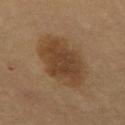<record>
  <biopsy_status>not biopsied; imaged during a skin examination</biopsy_status>
  <patient>
    <sex>male</sex>
    <age_approx>55</age_approx>
  </patient>
  <site>mid back</site>
  <lighting>cross-polarized</lighting>
  <image>
    <source>total-body photography crop</source>
    <field_of_view_mm>15</field_of_view_mm>
  </image>
  <lesion_size>
    <long_diameter_mm_approx>7.5</long_diameter_mm_approx>
  </lesion_size>
  <automated_metrics>
    <cielab_L>39</cielab_L>
    <cielab_a>16</cielab_a>
    <cielab_b>30</cielab_b>
    <vs_skin_darker_L>9.0</vs_skin_darker_L>
    <vs_skin_contrast_norm>8.0</vs_skin_contrast_norm>
    <nevus_likeness_0_100>95</nevus_likeness_0_100>
    <lesion_detection_confidence_0_100>100</lesion_detection_confidence_0_100>
  </automated_metrics>
</record>